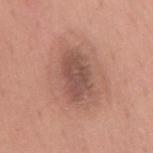No biopsy was performed on this lesion — it was imaged during a full skin examination and was not determined to be concerning. A female subject, aged around 65. A close-up tile cropped from a whole-body skin photograph, about 15 mm across. An algorithmic analysis of the crop reported a lesion color around L≈51 a*≈21 b*≈26 in CIELAB and about 11 CIELAB-L* units darker than the surrounding skin. And it measured a classifier nevus-likeness of about 0/100 and a lesion-detection confidence of about 100/100. On the mid back.